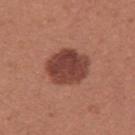Clinical impression: Recorded during total-body skin imaging; not selected for excision or biopsy. Background: A 15 mm crop from a total-body photograph taken for skin-cancer surveillance. A female patient, aged approximately 25. The lesion is on the right upper arm.